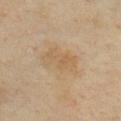Recorded during total-body skin imaging; not selected for excision or biopsy. A male patient aged around 40. On the front of the torso. A lesion tile, about 15 mm wide, cut from a 3D total-body photograph. Measured at roughly 5 mm in maximum diameter. Captured under cross-polarized illumination.